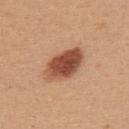A close-up tile cropped from a whole-body skin photograph, about 15 mm across.
Automated image analysis of the tile measured a footprint of about 14 mm², an eccentricity of roughly 0.8, and a symmetry-axis asymmetry near 0.15.
A female subject, in their mid-20s.
The lesion is located on the back.
This is a white-light tile.